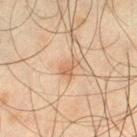Impression:
The lesion was tiled from a total-body skin photograph and was not biopsied.
Context:
A male subject in their mid- to late 40s. A 15 mm close-up tile from a total-body photography series done for melanoma screening. From the leg.